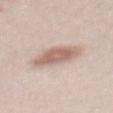Clinical impression:
Imaged during a routine full-body skin examination; the lesion was not biopsied and no histopathology is available.
Acquisition and patient details:
On the back. The patient is a male about 25 years old. A 15 mm crop from a total-body photograph taken for skin-cancer surveillance.